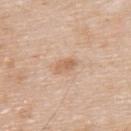{"biopsy_status": "not biopsied; imaged during a skin examination", "site": "upper back", "patient": {"sex": "male", "age_approx": 65}, "image": {"source": "total-body photography crop", "field_of_view_mm": 15}}